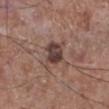biopsy status: no biopsy performed (imaged during a skin exam) | diameter: ≈3.5 mm | patient: male, aged around 65 | anatomic site: the right lower leg | image source: ~15 mm tile from a whole-body skin photo | lighting: white-light | image-analysis metrics: border irregularity of about 5 on a 0–10 scale, a color-variation rating of about 4.5/10, and peripheral color asymmetry of about 1.5; a detector confidence of about 100 out of 100 that the crop contains a lesion.